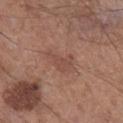Q: Is there a histopathology result?
A: no biopsy performed (imaged during a skin exam)
Q: What is the anatomic site?
A: the right lower leg
Q: What did automated image analysis measure?
A: a lesion area of about 7.5 mm², a shape eccentricity near 0.85, and a symmetry-axis asymmetry near 0.35; a border-irregularity rating of about 4/10 and a peripheral color-asymmetry measure near 0.5; a nevus-likeness score of about 0/100 and lesion-presence confidence of about 100/100
Q: Lesion size?
A: about 4.5 mm
Q: How was this image acquired?
A: ~15 mm crop, total-body skin-cancer survey
Q: Illumination type?
A: white-light illumination
Q: Who is the patient?
A: male, aged 53–57